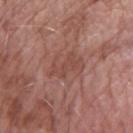{"biopsy_status": "not biopsied; imaged during a skin examination", "site": "left forearm", "lesion_size": {"long_diameter_mm_approx": 4.5}, "patient": {"sex": "female", "age_approx": 60}, "image": {"source": "total-body photography crop", "field_of_view_mm": 15}, "automated_metrics": {"area_mm2_approx": 7.5, "eccentricity": 0.85, "shape_asymmetry": 0.45}, "lighting": "white-light"}